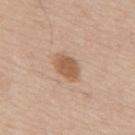workup = imaged on a skin check; not biopsied
automated lesion analysis = a lesion color around L≈57 a*≈20 b*≈33 in CIELAB and a normalized border contrast of about 8; a border-irregularity rating of about 2/10 and peripheral color asymmetry of about 0.5; a classifier nevus-likeness of about 90/100 and a lesion-detection confidence of about 100/100
size = about 3.5 mm
acquisition = 15 mm crop, total-body photography
patient = male, about 65 years old
tile lighting = white-light
site = the mid back